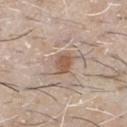Recorded during total-body skin imaging; not selected for excision or biopsy.
The recorded lesion diameter is about 2.5 mm.
The lesion is located on the chest.
A close-up tile cropped from a whole-body skin photograph, about 15 mm across.
Imaged with white-light lighting.
The lesion-visualizer software estimated a border-irregularity index near 2.5/10 and a peripheral color-asymmetry measure near 0.5. It also reported a nevus-likeness score of about 80/100 and a lesion-detection confidence of about 100/100.
A male patient approximately 65 years of age.